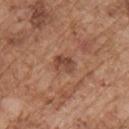Assessment:
Captured during whole-body skin photography for melanoma surveillance; the lesion was not biopsied.
Acquisition and patient details:
Located on the front of the torso. Cropped from a total-body skin-imaging series; the visible field is about 15 mm. The patient is a male about 75 years old. Automated tile analysis of the lesion measured border irregularity of about 2.5 on a 0–10 scale and a within-lesion color-variation index near 4.5/10. And it measured a classifier nevus-likeness of about 5/100. Longest diameter approximately 2.5 mm. Imaged with white-light lighting.